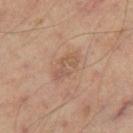This lesion was catalogued during total-body skin photography and was not selected for biopsy. An algorithmic analysis of the crop reported an eccentricity of roughly 0.85. This image is a 15 mm lesion crop taken from a total-body photograph. The lesion's longest dimension is about 4 mm. On the leg. This is a cross-polarized tile. A male subject aged 48 to 52.